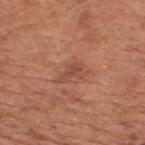{"automated_metrics": {"area_mm2_approx": 4.5, "eccentricity": 0.85, "shape_asymmetry": 0.55}, "lighting": "white-light", "patient": {"sex": "male", "age_approx": 65}, "site": "upper back", "image": {"source": "total-body photography crop", "field_of_view_mm": 15}, "lesion_size": {"long_diameter_mm_approx": 3.5}}